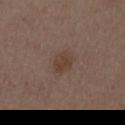biopsy_status: not biopsied; imaged during a skin examination
lesion_size:
  long_diameter_mm_approx: 3.0
image:
  source: total-body photography crop
  field_of_view_mm: 15
site: abdomen
patient:
  sex: male
  age_approx: 50